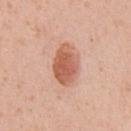Part of a total-body skin-imaging series; this lesion was reviewed on a skin check and was not flagged for biopsy. Measured at roughly 5 mm in maximum diameter. Cropped from a whole-body photographic skin survey; the tile spans about 15 mm. The patient is a male aged 53 to 57. Captured under white-light illumination. From the abdomen.